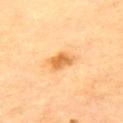Notes:
• workup: imaged on a skin check; not biopsied
• body site: the upper back
• subject: male, aged approximately 85 or older
• imaging modality: ~15 mm tile from a whole-body skin photo